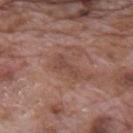This lesion was catalogued during total-body skin photography and was not selected for biopsy.
Cropped from a total-body skin-imaging series; the visible field is about 15 mm.
The lesion is located on the mid back.
Captured under white-light illumination.
A male subject, aged 68–72.
The lesion's longest dimension is about 3.5 mm.